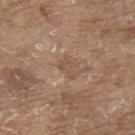Q: What kind of image is this?
A: ~15 mm crop, total-body skin-cancer survey
Q: Automated lesion metrics?
A: a footprint of about 3 mm², a shape eccentricity near 0.85, and a shape-asymmetry score of about 0.5 (0 = symmetric); a lesion color around L≈51 a*≈17 b*≈29 in CIELAB, roughly 7 lightness units darker than nearby skin, and a normalized border contrast of about 5; a border-irregularity rating of about 5/10, internal color variation of about 1 on a 0–10 scale, and peripheral color asymmetry of about 0.5
Q: Lesion size?
A: ≈2.5 mm
Q: What is the anatomic site?
A: the upper back
Q: Patient demographics?
A: male, roughly 80 years of age
Q: What lighting was used for the tile?
A: white-light illumination